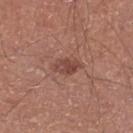Imaged during a routine full-body skin examination; the lesion was not biopsied and no histopathology is available.
Located on the right lower leg.
A male subject aged 68 to 72.
An algorithmic analysis of the crop reported a lesion area of about 5.5 mm², a shape eccentricity near 0.8, and a symmetry-axis asymmetry near 0.2.
This image is a 15 mm lesion crop taken from a total-body photograph.